The total-body-photography lesion software estimated an eccentricity of roughly 0.4 and a symmetry-axis asymmetry near 0.15. It also reported a lesion–skin lightness drop of about 11. It also reported a border-irregularity rating of about 1.5/10 and internal color variation of about 2.5 on a 0–10 scale. The software also gave an automated nevus-likeness rating near 85 out of 100 and a detector confidence of about 100 out of 100 that the crop contains a lesion. This image is a 15 mm lesion crop taken from a total-body photograph. A female patient, aged around 25. From the leg. Captured under white-light illumination: 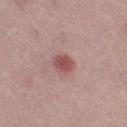diagnosis = a dysplastic (Clark) nevus